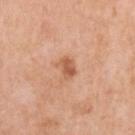The lesion was tiled from a total-body skin photograph and was not biopsied.
This is a white-light tile.
A female subject aged 53 to 57.
On the left upper arm.
Approximately 2.5 mm at its widest.
A lesion tile, about 15 mm wide, cut from a 3D total-body photograph.
Automated image analysis of the tile measured a footprint of about 4 mm², an outline eccentricity of about 0.75 (0 = round, 1 = elongated), and a shape-asymmetry score of about 0.25 (0 = symmetric). And it measured a classifier nevus-likeness of about 25/100 and lesion-presence confidence of about 100/100.